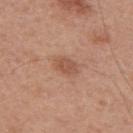<record>
<biopsy_status>not biopsied; imaged during a skin examination</biopsy_status>
<patient>
  <sex>male</sex>
  <age_approx>30</age_approx>
</patient>
<image>
  <source>total-body photography crop</source>
  <field_of_view_mm>15</field_of_view_mm>
</image>
<site>upper back</site>
<lighting>white-light</lighting>
</record>